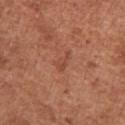The lesion was tiled from a total-body skin photograph and was not biopsied. A lesion tile, about 15 mm wide, cut from a 3D total-body photograph. From the right upper arm. Measured at roughly 2.5 mm in maximum diameter. Automated image analysis of the tile measured border irregularity of about 5.5 on a 0–10 scale, internal color variation of about 0 on a 0–10 scale, and peripheral color asymmetry of about 0. It also reported an automated nevus-likeness rating near 0 out of 100. The subject is a female aged around 50.